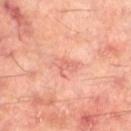The lesion was photographed on a routine skin check and not biopsied; there is no pathology result.
Measured at roughly 3 mm in maximum diameter.
Automated tile analysis of the lesion measured an area of roughly 4 mm² and a shape-asymmetry score of about 0.3 (0 = symmetric). The analysis additionally found border irregularity of about 3.5 on a 0–10 scale and a within-lesion color-variation index near 2/10.
A male subject aged around 60.
A close-up tile cropped from a whole-body skin photograph, about 15 mm across.
This is a cross-polarized tile.
Located on the right thigh.